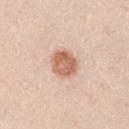Recorded during total-body skin imaging; not selected for excision or biopsy. The tile uses white-light illumination. A male patient aged 43–47. The total-body-photography lesion software estimated an area of roughly 9 mm², a shape eccentricity near 0.5, and a symmetry-axis asymmetry near 0.15. The software also gave border irregularity of about 1 on a 0–10 scale and a peripheral color-asymmetry measure near 1. The analysis additionally found a detector confidence of about 100 out of 100 that the crop contains a lesion. Located on the arm. A lesion tile, about 15 mm wide, cut from a 3D total-body photograph. Approximately 3.5 mm at its widest.